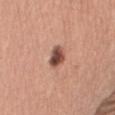• follow-up: total-body-photography surveillance lesion; no biopsy
• diameter: ≈3 mm
• lighting: white-light
• imaging modality: ~15 mm tile from a whole-body skin photo
• subject: female, aged around 55
• location: the right upper arm